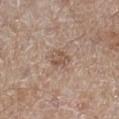Clinical impression: Captured during whole-body skin photography for melanoma surveillance; the lesion was not biopsied. Image and clinical context: On the right lower leg. The subject is a male aged 63 to 67. Captured under white-light illumination. Approximately 2.5 mm at its widest. Cropped from a total-body skin-imaging series; the visible field is about 15 mm.